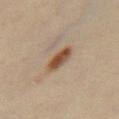lighting = cross-polarized illumination | site = the mid back | image = 15 mm crop, total-body photography | lesion diameter = about 3.5 mm | subject = male, aged around 50.